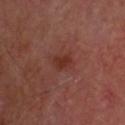Notes:
– image — ~15 mm tile from a whole-body skin photo
– illumination — cross-polarized illumination
– anatomic site — the head or neck
– lesion size — ~2.5 mm (longest diameter)
– subject — male, aged approximately 60